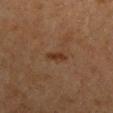Impression:
Imaged during a routine full-body skin examination; the lesion was not biopsied and no histopathology is available.
Acquisition and patient details:
The lesion is on the left upper arm. The lesion-visualizer software estimated an outline eccentricity of about 0.9 (0 = round, 1 = elongated) and a symmetry-axis asymmetry near 0.35. And it measured a border-irregularity index near 3.5/10, a within-lesion color-variation index near 0/10, and a peripheral color-asymmetry measure near 0. The software also gave an automated nevus-likeness rating near 65 out of 100 and a detector confidence of about 100 out of 100 that the crop contains a lesion. The tile uses cross-polarized illumination. A roughly 15 mm field-of-view crop from a total-body skin photograph. The subject is a male aged around 65.